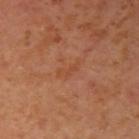Q: Is there a histopathology result?
A: no biopsy performed (imaged during a skin exam)
Q: Lesion location?
A: the right upper arm
Q: What are the patient's age and sex?
A: female, aged around 40
Q: How was this image acquired?
A: total-body-photography crop, ~15 mm field of view